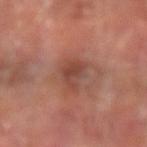follow-up: no biopsy performed (imaged during a skin exam)
image: total-body-photography crop, ~15 mm field of view
site: the right forearm
lesion diameter: ~4.5 mm (longest diameter)
lighting: cross-polarized illumination
image-analysis metrics: a mean CIELAB color near L≈43 a*≈23 b*≈26, about 9 CIELAB-L* units darker than the surrounding skin, and a normalized lesion–skin contrast near 6.5; border irregularity of about 4 on a 0–10 scale, internal color variation of about 3.5 on a 0–10 scale, and a peripheral color-asymmetry measure near 1; an automated nevus-likeness rating near 0 out of 100
patient: male, roughly 65 years of age